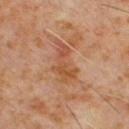Clinical summary: Longest diameter approximately 5.5 mm. The subject is a male aged approximately 60. A close-up tile cropped from a whole-body skin photograph, about 15 mm across. The lesion is located on the chest. Imaged with cross-polarized lighting.